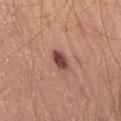Imaged during a routine full-body skin examination; the lesion was not biopsied and no histopathology is available.
An algorithmic analysis of the crop reported an area of roughly 4.5 mm², a shape eccentricity near 0.75, and a shape-asymmetry score of about 0.2 (0 = symmetric). The analysis additionally found a mean CIELAB color near L≈43 a*≈26 b*≈21, roughly 15 lightness units darker than nearby skin, and a lesion-to-skin contrast of about 12 (normalized; higher = more distinct). It also reported a detector confidence of about 100 out of 100 that the crop contains a lesion.
Approximately 3 mm at its widest.
A male subject, aged approximately 65.
On the right thigh.
A 15 mm crop from a total-body photograph taken for skin-cancer surveillance.
Imaged with cross-polarized lighting.